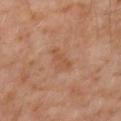Recorded during total-body skin imaging; not selected for excision or biopsy.
About 4 mm across.
The patient is a male aged 58 to 62.
This image is a 15 mm lesion crop taken from a total-body photograph.
From the mid back.